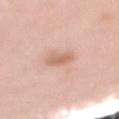<record>
  <biopsy_status>not biopsied; imaged during a skin examination</biopsy_status>
  <image>
    <source>total-body photography crop</source>
    <field_of_view_mm>15</field_of_view_mm>
  </image>
  <lesion_size>
    <long_diameter_mm_approx>2.5</long_diameter_mm_approx>
  </lesion_size>
  <patient>
    <sex>female</sex>
    <age_approx>70</age_approx>
  </patient>
  <lighting>white-light</lighting>
  <automated_metrics>
    <area_mm2_approx>4.0</area_mm2_approx>
    <eccentricity>0.7</eccentricity>
    <shape_asymmetry>0.3</shape_asymmetry>
    <vs_skin_darker_L>10.0</vs_skin_darker_L>
    <vs_skin_contrast_norm>6.5</vs_skin_contrast_norm>
    <border_irregularity_0_10>3.0</border_irregularity_0_10>
    <color_variation_0_10>2.0</color_variation_0_10>
    <peripheral_color_asymmetry>0.5</peripheral_color_asymmetry>
    <nevus_likeness_0_100>15</nevus_likeness_0_100>
    <lesion_detection_confidence_0_100>100</lesion_detection_confidence_0_100>
  </automated_metrics>
  <site>mid back</site>
</record>